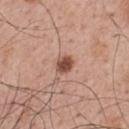TBP lesion metrics: an area of roughly 4 mm², an outline eccentricity of about 0.55 (0 = round, 1 = elongated), and a symmetry-axis asymmetry near 0.15; a mean CIELAB color near L≈50 a*≈22 b*≈28, a lesion–skin lightness drop of about 15, and a normalized border contrast of about 10; a color-variation rating of about 4/10; an automated nevus-likeness rating near 95 out of 100 | image: ~15 mm crop, total-body skin-cancer survey | anatomic site: the upper back | tile lighting: white-light illumination | subject: male, in their mid- to late 60s | lesion size: about 2.5 mm.